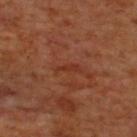  biopsy_status: not biopsied; imaged during a skin examination
  lighting: cross-polarized
  patient:
    sex: male
    age_approx: 70
  lesion_size:
    long_diameter_mm_approx: 3.0
  image:
    source: total-body photography crop
    field_of_view_mm: 15
  site: back